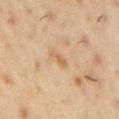image:
  source: total-body photography crop
  field_of_view_mm: 15
lesion_size:
  long_diameter_mm_approx: 2.5
patient:
  sex: male
  age_approx: 55
site: right upper arm
lighting: cross-polarized